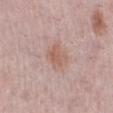Impression:
No biopsy was performed on this lesion — it was imaged during a full skin examination and was not determined to be concerning.
Clinical summary:
The lesion's longest dimension is about 2.5 mm. A female patient, aged around 65. On the left leg. A lesion tile, about 15 mm wide, cut from a 3D total-body photograph. Imaged with white-light lighting.